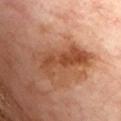Findings:
- biopsy status: no biopsy performed (imaged during a skin exam)
- patient: male, about 70 years old
- tile lighting: cross-polarized illumination
- lesion size: ≈6.5 mm
- anatomic site: the chest
- image source: total-body-photography crop, ~15 mm field of view